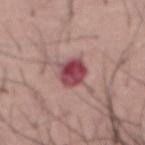Impression: Recorded during total-body skin imaging; not selected for excision or biopsy.